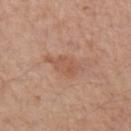{
  "biopsy_status": "not biopsied; imaged during a skin examination",
  "automated_metrics": {
    "nevus_likeness_0_100": 0,
    "lesion_detection_confidence_0_100": 100
  },
  "image": {
    "source": "total-body photography crop",
    "field_of_view_mm": 15
  },
  "site": "left forearm",
  "patient": {
    "sex": "male",
    "age_approx": 60
  },
  "lighting": "white-light"
}